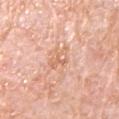Q: Is there a histopathology result?
A: total-body-photography surveillance lesion; no biopsy
Q: What lighting was used for the tile?
A: white-light
Q: Lesion size?
A: ≈3.5 mm
Q: Who is the patient?
A: male, aged 78 to 82
Q: Lesion location?
A: the left upper arm
Q: What is the imaging modality?
A: ~15 mm tile from a whole-body skin photo